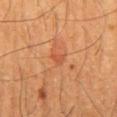notes = catalogued during a skin exam; not biopsied | anatomic site = the mid back | tile lighting = cross-polarized illumination | subject = male, roughly 60 years of age | image source = total-body-photography crop, ~15 mm field of view | lesion size = ≈2 mm.